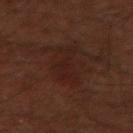No biopsy was performed on this lesion — it was imaged during a full skin examination and was not determined to be concerning. The lesion is located on the left forearm. A male subject aged around 60. The total-body-photography lesion software estimated a footprint of about 8.5 mm² and a symmetry-axis asymmetry near 0.55. Measured at roughly 5 mm in maximum diameter. A roughly 15 mm field-of-view crop from a total-body skin photograph.